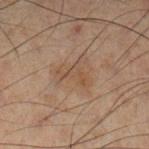| field | value |
|---|---|
| follow-up | total-body-photography surveillance lesion; no biopsy |
| body site | the left lower leg |
| subject | male, in their 50s |
| lesion diameter | ≈3.5 mm |
| tile lighting | cross-polarized |
| imaging modality | 15 mm crop, total-body photography |